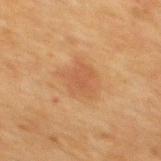notes — no biopsy performed (imaged during a skin exam) | patient — male, aged approximately 50 | imaging modality — 15 mm crop, total-body photography | anatomic site — the mid back | automated metrics — a classifier nevus-likeness of about 5/100 and lesion-presence confidence of about 100/100 | lesion size — about 4.5 mm.